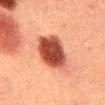Clinical impression:
The lesion was tiled from a total-body skin photograph and was not biopsied.
Image and clinical context:
On the mid back. A male patient aged 38 to 42. A 15 mm close-up extracted from a 3D total-body photography capture. Imaged with cross-polarized lighting. An algorithmic analysis of the crop reported an area of roughly 15 mm² and a shape eccentricity near 0.8.